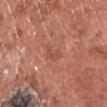subject: male, aged 73–77; image source: 15 mm crop, total-body photography; site: the chest.